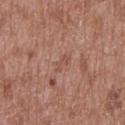Findings:
* biopsy status: imaged on a skin check; not biopsied
* size: ~2.5 mm (longest diameter)
* subject: male, aged around 50
* lighting: white-light illumination
* anatomic site: the mid back
* imaging modality: total-body-photography crop, ~15 mm field of view